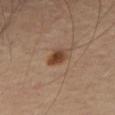biopsy status — no biopsy performed (imaged during a skin exam).